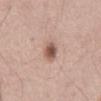Assessment:
Part of a total-body skin-imaging series; this lesion was reviewed on a skin check and was not flagged for biopsy.
Background:
The tile uses white-light illumination. Measured at roughly 3 mm in maximum diameter. The lesion is located on the abdomen. The subject is a male aged approximately 55. A 15 mm close-up tile from a total-body photography series done for melanoma screening. An algorithmic analysis of the crop reported an eccentricity of roughly 0.8 and a shape-asymmetry score of about 0.2 (0 = symmetric). It also reported a border-irregularity index near 2/10, a within-lesion color-variation index near 4.5/10, and a peripheral color-asymmetry measure near 1.5.